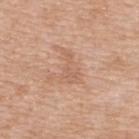| key | value |
|---|---|
| workup | no biopsy performed (imaged during a skin exam) |
| patient | female, approximately 55 years of age |
| TBP lesion metrics | a lesion–skin lightness drop of about 7 and a lesion-to-skin contrast of about 4.5 (normalized; higher = more distinct); a color-variation rating of about 1/10 and a peripheral color-asymmetry measure near 0.5 |
| lighting | white-light |
| acquisition | total-body-photography crop, ~15 mm field of view |
| size | ≈4 mm |
| site | the upper back |